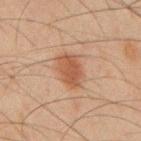Impression: Imaged during a routine full-body skin examination; the lesion was not biopsied and no histopathology is available. Image and clinical context: A 15 mm crop from a total-body photograph taken for skin-cancer surveillance. The recorded lesion diameter is about 4 mm. Located on the mid back. A male patient approximately 45 years of age. Captured under cross-polarized illumination.